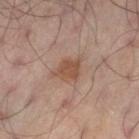Notes:
- notes — no biopsy performed (imaged during a skin exam)
- imaging modality — ~15 mm tile from a whole-body skin photo
- lesion diameter — about 3 mm
- tile lighting — cross-polarized illumination
- subject — male, in their mid- to late 60s
- body site — the left thigh